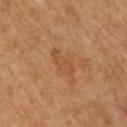Imaged during a routine full-body skin examination; the lesion was not biopsied and no histopathology is available. The lesion is located on the back. An algorithmic analysis of the crop reported an area of roughly 5.5 mm², a shape eccentricity near 0.9, and two-axis asymmetry of about 0.3. The analysis additionally found a lesion–skin lightness drop of about 6. And it measured a border-irregularity rating of about 4/10, a color-variation rating of about 1.5/10, and a peripheral color-asymmetry measure near 0.5. And it measured an automated nevus-likeness rating near 0 out of 100 and a detector confidence of about 100 out of 100 that the crop contains a lesion. Imaged with cross-polarized lighting. Measured at roughly 4 mm in maximum diameter. The patient is roughly 60 years of age. This image is a 15 mm lesion crop taken from a total-body photograph.